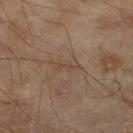Impression: Captured during whole-body skin photography for melanoma surveillance; the lesion was not biopsied. Acquisition and patient details: A male patient aged around 45. The tile uses cross-polarized illumination. A 15 mm close-up tile from a total-body photography series done for melanoma screening. Approximately 3 mm at its widest. The lesion is located on the left lower leg. The lesion-visualizer software estimated an area of roughly 2.5 mm² and a symmetry-axis asymmetry near 0.55. And it measured a lesion color around L≈34 a*≈13 b*≈23 in CIELAB and roughly 4 lightness units darker than nearby skin.